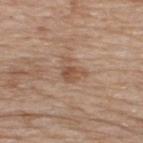{"biopsy_status": "not biopsied; imaged during a skin examination", "patient": {"sex": "male", "age_approx": 65}, "site": "upper back", "image": {"source": "total-body photography crop", "field_of_view_mm": 15}}